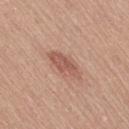Clinical impression:
The lesion was tiled from a total-body skin photograph and was not biopsied.
Clinical summary:
Located on the right thigh. The patient is a female approximately 55 years of age. Approximately 4 mm at its widest. Cropped from a whole-body photographic skin survey; the tile spans about 15 mm.